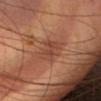{
  "biopsy_status": "not biopsied; imaged during a skin examination",
  "automated_metrics": {
    "area_mm2_approx": 7.5,
    "eccentricity": 0.8,
    "shape_asymmetry": 0.25,
    "vs_skin_darker_L": 7.0,
    "vs_skin_contrast_norm": 5.5
  },
  "lighting": "cross-polarized",
  "site": "head or neck",
  "image": {
    "source": "total-body photography crop",
    "field_of_view_mm": 15
  },
  "patient": {
    "sex": "female",
    "age_approx": 40
  },
  "lesion_size": {
    "long_diameter_mm_approx": 4.0
  }
}